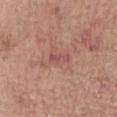| key | value |
|---|---|
| workup | no biopsy performed (imaged during a skin exam) |
| acquisition | ~15 mm crop, total-body skin-cancer survey |
| patient | female, aged approximately 65 |
| body site | the right forearm |
| lighting | white-light |